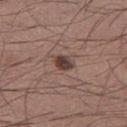Recorded during total-body skin imaging; not selected for excision or biopsy.
The subject is a male aged 33 to 37.
From the left lower leg.
Cropped from a total-body skin-imaging series; the visible field is about 15 mm.